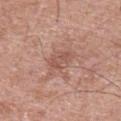Assessment:
The lesion was tiled from a total-body skin photograph and was not biopsied.
Background:
On the chest. Approximately 3 mm at its widest. The patient is a male about 70 years old. The total-body-photography lesion software estimated an area of roughly 4 mm² and an outline eccentricity of about 0.85 (0 = round, 1 = elongated). It also reported an average lesion color of about L≈53 a*≈23 b*≈26 (CIELAB), roughly 8 lightness units darker than nearby skin, and a normalized lesion–skin contrast near 5.5. The analysis additionally found a peripheral color-asymmetry measure near 0.5. The software also gave a classifier nevus-likeness of about 0/100. Captured under white-light illumination. A roughly 15 mm field-of-view crop from a total-body skin photograph.